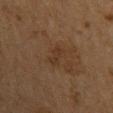This image is a 15 mm lesion crop taken from a total-body photograph.
A male patient, in their 60s.
The recorded lesion diameter is about 2.5 mm.